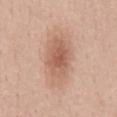biopsy_status: not biopsied; imaged during a skin examination
automated_metrics:
  area_mm2_approx: 17.0
  eccentricity: 0.85
  shape_asymmetry: 0.2
  border_irregularity_0_10: 2.5
  color_variation_0_10: 4.0
lighting: white-light
site: abdomen
image:
  source: total-body photography crop
  field_of_view_mm: 15
patient:
  sex: male
  age_approx: 50
lesion_size:
  long_diameter_mm_approx: 6.5